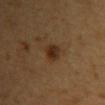Captured under cross-polarized illumination.
The lesion is on the upper back.
A 15 mm crop from a total-body photograph taken for skin-cancer surveillance.
The patient is a female roughly 30 years of age.